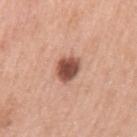This lesion was catalogued during total-body skin photography and was not selected for biopsy. A male subject roughly 60 years of age. On the left upper arm. Approximately 3 mm at its widest. A 15 mm crop from a total-body photograph taken for skin-cancer surveillance.